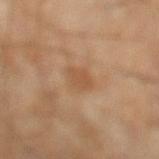biopsy_status: not biopsied; imaged during a skin examination
lesion_size:
  long_diameter_mm_approx: 3.0
automated_metrics:
  cielab_L: 52
  cielab_a: 20
  cielab_b: 34
  vs_skin_darker_L: 7.0
  vs_skin_contrast_norm: 5.5
  nevus_likeness_0_100: 0
  lesion_detection_confidence_0_100: 100
lighting: cross-polarized
image:
  source: total-body photography crop
  field_of_view_mm: 15
patient:
  age_approx: 55
site: left lower leg